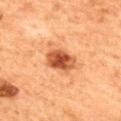Assessment:
This lesion was catalogued during total-body skin photography and was not selected for biopsy.
Acquisition and patient details:
The patient is a female about 60 years old. The lesion is on the upper back. The lesion's longest dimension is about 4 mm. The lesion-visualizer software estimated a lesion area of about 9 mm² and two-axis asymmetry of about 0.15. And it measured a detector confidence of about 100 out of 100 that the crop contains a lesion. Captured under cross-polarized illumination. Cropped from a total-body skin-imaging series; the visible field is about 15 mm.